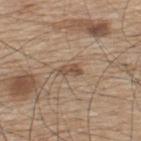The lesion was photographed on a routine skin check and not biopsied; there is no pathology result.
On the upper back.
The patient is a male about 75 years old.
A 15 mm crop from a total-body photograph taken for skin-cancer surveillance.
About 3 mm across.